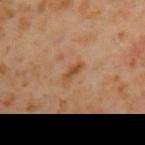biopsy status: total-body-photography surveillance lesion; no biopsy
subject: male, aged around 60
location: the left upper arm
lighting: cross-polarized
acquisition: ~15 mm tile from a whole-body skin photo